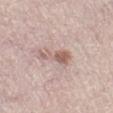Captured during whole-body skin photography for melanoma surveillance; the lesion was not biopsied. A female subject, in their mid- to late 60s. Captured under white-light illumination. The total-body-photography lesion software estimated an outline eccentricity of about 0.9 (0 = round, 1 = elongated) and a symmetry-axis asymmetry near 0.55. The analysis additionally found a border-irregularity index near 6.5/10 and internal color variation of about 2 on a 0–10 scale. And it measured a classifier nevus-likeness of about 30/100 and lesion-presence confidence of about 100/100. Located on the left lower leg. A 15 mm close-up extracted from a 3D total-body photography capture.